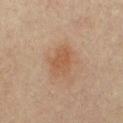Findings:
• follow-up · total-body-photography surveillance lesion; no biopsy
• subject · male, aged 43 to 47
• body site · the chest
• image · total-body-photography crop, ~15 mm field of view
• lesion diameter · ≈4 mm
• lighting · cross-polarized